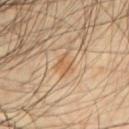Recorded during total-body skin imaging; not selected for excision or biopsy. This image is a 15 mm lesion crop taken from a total-body photograph. Approximately 2.5 mm at its widest. From the chest. The subject is a male roughly 60 years of age.